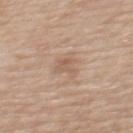Q: Was this lesion biopsied?
A: no biopsy performed (imaged during a skin exam)
Q: Automated lesion metrics?
A: an area of roughly 5 mm², an outline eccentricity of about 0.8 (0 = round, 1 = elongated), and a symmetry-axis asymmetry near 0.4; border irregularity of about 5 on a 0–10 scale, a color-variation rating of about 2.5/10, and peripheral color asymmetry of about 1; a classifier nevus-likeness of about 0/100 and a lesion-detection confidence of about 100/100
Q: Where on the body is the lesion?
A: the upper back
Q: What kind of image is this?
A: ~15 mm tile from a whole-body skin photo
Q: What are the patient's age and sex?
A: female, aged 63–67